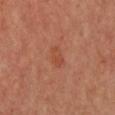Recorded during total-body skin imaging; not selected for excision or biopsy. The subject is a female approximately 65 years of age. The lesion is located on the chest. A roughly 15 mm field-of-view crop from a total-body skin photograph. The recorded lesion diameter is about 2.5 mm. Automated image analysis of the tile measured an average lesion color of about L≈45 a*≈26 b*≈32 (CIELAB), about 6 CIELAB-L* units darker than the surrounding skin, and a normalized border contrast of about 5.